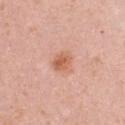workup — no biopsy performed (imaged during a skin exam)
anatomic site — the chest
automated metrics — a normalized border contrast of about 7; border irregularity of about 2 on a 0–10 scale and peripheral color asymmetry of about 1.5; an automated nevus-likeness rating near 75 out of 100 and lesion-presence confidence of about 100/100
diameter — ≈2.5 mm
lighting — white-light
image — ~15 mm tile from a whole-body skin photo
patient — female, in their 30s